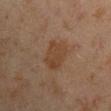The lesion was tiled from a total-body skin photograph and was not biopsied.
Imaged with cross-polarized lighting.
The recorded lesion diameter is about 4.5 mm.
A roughly 15 mm field-of-view crop from a total-body skin photograph.
Located on the right upper arm.
A male subject, in their mid- to late 40s.